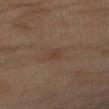Captured during whole-body skin photography for melanoma surveillance; the lesion was not biopsied.
Longest diameter approximately 2.5 mm.
A female patient, aged 58–62.
The tile uses cross-polarized illumination.
A close-up tile cropped from a whole-body skin photograph, about 15 mm across.
The total-body-photography lesion software estimated a footprint of about 3 mm², an outline eccentricity of about 0.8 (0 = round, 1 = elongated), and two-axis asymmetry of about 0.2. It also reported an average lesion color of about L≈35 a*≈14 b*≈24 (CIELAB) and roughly 4 lightness units darker than nearby skin.
From the arm.